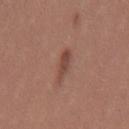Impression:
This lesion was catalogued during total-body skin photography and was not selected for biopsy.
Background:
A male subject in their 30s. A 15 mm close-up tile from a total-body photography series done for melanoma screening. The lesion-visualizer software estimated an area of roughly 4 mm², an outline eccentricity of about 0.9 (0 = round, 1 = elongated), and a symmetry-axis asymmetry near 0.3. And it measured a lesion color around L≈45 a*≈22 b*≈26 in CIELAB and a lesion–skin lightness drop of about 9. The software also gave a nevus-likeness score of about 75/100. This is a white-light tile. Located on the mid back. Approximately 3.5 mm at its widest.